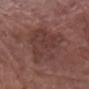| feature | finding |
|---|---|
| biopsy status | no biopsy performed (imaged during a skin exam) |
| illumination | white-light |
| image | ~15 mm crop, total-body skin-cancer survey |
| subject | female, aged 68–72 |
| automated metrics | a border-irregularity index near 3.5/10, internal color variation of about 2.5 on a 0–10 scale, and peripheral color asymmetry of about 1; an automated nevus-likeness rating near 0 out of 100 and a detector confidence of about 100 out of 100 that the crop contains a lesion |
| lesion size | ~7.5 mm (longest diameter) |
| anatomic site | the right forearm |